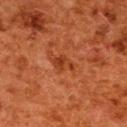No biopsy was performed on this lesion — it was imaged during a full skin examination and was not determined to be concerning.
The lesion is on the back.
The subject is a female in their 50s.
The tile uses cross-polarized illumination.
Measured at roughly 2.5 mm in maximum diameter.
A 15 mm close-up extracted from a 3D total-body photography capture.
An algorithmic analysis of the crop reported an area of roughly 3.5 mm² and an eccentricity of roughly 0.75. And it measured border irregularity of about 4.5 on a 0–10 scale, a within-lesion color-variation index near 1.5/10, and peripheral color asymmetry of about 0.5. The analysis additionally found a nevus-likeness score of about 0/100 and a detector confidence of about 100 out of 100 that the crop contains a lesion.